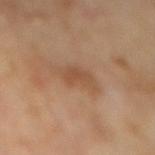Recorded during total-body skin imaging; not selected for excision or biopsy. The lesion is located on the back. A 15 mm close-up tile from a total-body photography series done for melanoma screening. Imaged with cross-polarized lighting. Approximately 4.5 mm at its widest. A male subject in their 70s.